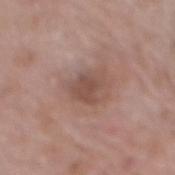follow-up: catalogued during a skin exam; not biopsied | site: the back | subject: male, aged approximately 70 | image source: total-body-photography crop, ~15 mm field of view.